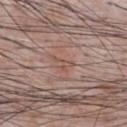Notes:
• follow-up · catalogued during a skin exam; not biopsied
• location · the chest
• lesion size · ~3 mm (longest diameter)
• subject · male, aged 58–62
• image · ~15 mm crop, total-body skin-cancer survey
• image-analysis metrics · an average lesion color of about L≈52 a*≈19 b*≈25 (CIELAB) and a normalized lesion–skin contrast near 5; radial color variation of about 0; an automated nevus-likeness rating near 0 out of 100 and a lesion-detection confidence of about 80/100